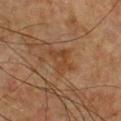workup: total-body-photography surveillance lesion; no biopsy | site: the chest | size: ≈3.5 mm | acquisition: ~15 mm tile from a whole-body skin photo | patient: male, aged 48–52.